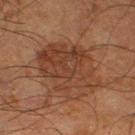workup — catalogued during a skin exam; not biopsied | anatomic site — the left lower leg | lighting — cross-polarized | lesion diameter — about 7 mm | patient — male, in their mid- to late 60s | image source — total-body-photography crop, ~15 mm field of view.